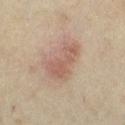Impression: The lesion was photographed on a routine skin check and not biopsied; there is no pathology result.